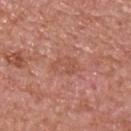Q: Was this lesion biopsied?
A: total-body-photography surveillance lesion; no biopsy
Q: What are the patient's age and sex?
A: male, in their mid- to late 60s
Q: Lesion location?
A: the back
Q: How was this image acquired?
A: ~15 mm crop, total-body skin-cancer survey
Q: What lighting was used for the tile?
A: white-light illumination